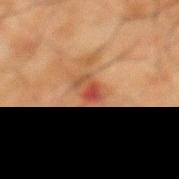Clinical impression: Recorded during total-body skin imaging; not selected for excision or biopsy. Background: A male patient, aged around 65. Imaged with cross-polarized lighting. Longest diameter approximately 3 mm. A roughly 15 mm field-of-view crop from a total-body skin photograph. On the mid back.